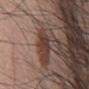Clinical impression:
Imaged during a routine full-body skin examination; the lesion was not biopsied and no histopathology is available.
Background:
The lesion is located on the back. A roughly 15 mm field-of-view crop from a total-body skin photograph. Automated image analysis of the tile measured a lesion area of about 3.5 mm², an eccentricity of roughly 0.7, and two-axis asymmetry of about 0.25. The software also gave a lesion-to-skin contrast of about 8 (normalized; higher = more distinct). The analysis additionally found a classifier nevus-likeness of about 100/100 and lesion-presence confidence of about 95/100. A male subject about 70 years old.